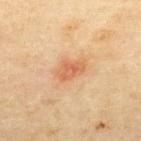Imaged during a routine full-body skin examination; the lesion was not biopsied and no histopathology is available. Automated image analysis of the tile measured an automated nevus-likeness rating near 35 out of 100 and lesion-presence confidence of about 100/100. On the back. The patient is a male in their mid-40s. A region of skin cropped from a whole-body photographic capture, roughly 15 mm wide. The tile uses cross-polarized illumination. Longest diameter approximately 3.5 mm.